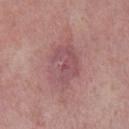Case summary:
- location — the left lower leg
- image — 15 mm crop, total-body photography
- subject — female, aged 38–42